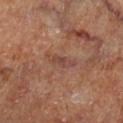<tbp_lesion>
<site>right lower leg</site>
<patient>
  <sex>male</sex>
  <age_approx>60</age_approx>
</patient>
<lesion_size>
  <long_diameter_mm_approx>4.0</long_diameter_mm_approx>
</lesion_size>
<image>
  <source>total-body photography crop</source>
  <field_of_view_mm>15</field_of_view_mm>
</image>
</tbp_lesion>